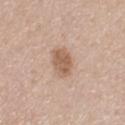This lesion was catalogued during total-body skin photography and was not selected for biopsy. Automated image analysis of the tile measured a lesion area of about 8 mm², an eccentricity of roughly 0.7, and a symmetry-axis asymmetry near 0.15. The analysis additionally found a lesion color around L≈59 a*≈18 b*≈30 in CIELAB, a lesion–skin lightness drop of about 11, and a lesion-to-skin contrast of about 7.5 (normalized; higher = more distinct). The analysis additionally found a border-irregularity index near 1.5/10, a color-variation rating of about 3/10, and a peripheral color-asymmetry measure near 1. The software also gave a lesion-detection confidence of about 100/100. From the upper back. A male subject, aged 38 to 42. A 15 mm close-up tile from a total-body photography series done for melanoma screening.